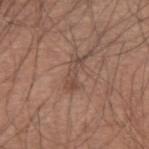| feature | finding |
|---|---|
| anatomic site | the arm |
| patient | male, aged around 45 |
| illumination | white-light illumination |
| image source | total-body-photography crop, ~15 mm field of view |